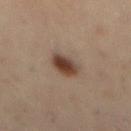biopsy_status: not biopsied; imaged during a skin examination
image:
  source: total-body photography crop
  field_of_view_mm: 15
lighting: cross-polarized
site: mid back
lesion_size:
  long_diameter_mm_approx: 3.5
patient:
  sex: male
  age_approx: 55